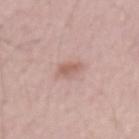A region of skin cropped from a whole-body photographic capture, roughly 15 mm wide.
Longest diameter approximately 2.5 mm.
The tile uses white-light illumination.
A male patient, about 55 years old.